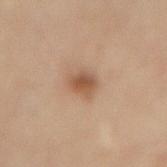Image and clinical context: Automated tile analysis of the lesion measured a footprint of about 5 mm², an outline eccentricity of about 0.6 (0 = round, 1 = elongated), and two-axis asymmetry of about 0.25. The analysis additionally found a peripheral color-asymmetry measure near 1. It also reported a classifier nevus-likeness of about 95/100 and a lesion-detection confidence of about 100/100. The recorded lesion diameter is about 2.5 mm. This is a cross-polarized tile. The lesion is on the mid back. The patient is a female roughly 60 years of age. A region of skin cropped from a whole-body photographic capture, roughly 15 mm wide.